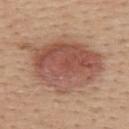Imaged during a routine full-body skin examination; the lesion was not biopsied and no histopathology is available. Longest diameter approximately 9 mm. Captured under white-light illumination. A female subject aged 43 to 47. Automated tile analysis of the lesion measured a lesion area of about 44 mm² and an outline eccentricity of about 0.65 (0 = round, 1 = elongated). The analysis additionally found lesion-presence confidence of about 100/100. The lesion is on the upper back. A 15 mm crop from a total-body photograph taken for skin-cancer surveillance.